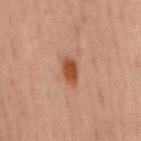Findings:
- follow-up — imaged on a skin check; not biopsied
- size — ≈3.5 mm
- illumination — cross-polarized
- body site — the chest
- image — 15 mm crop, total-body photography
- patient — male, aged around 70